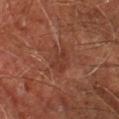biopsy status — no biopsy performed (imaged during a skin exam)
anatomic site — the leg
automated metrics — a shape eccentricity near 0.5 and two-axis asymmetry of about 0.2; a lesion color around L≈36 a*≈23 b*≈27 in CIELAB and roughly 5 lightness units darker than nearby skin; a detector confidence of about 100 out of 100 that the crop contains a lesion
diameter — ~3.5 mm (longest diameter)
image source — ~15 mm crop, total-body skin-cancer survey
patient — male, aged approximately 60
tile lighting — cross-polarized